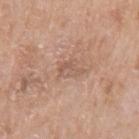Clinical impression:
No biopsy was performed on this lesion — it was imaged during a full skin examination and was not determined to be concerning.